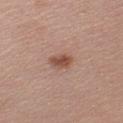Q: Was this lesion biopsied?
A: imaged on a skin check; not biopsied
Q: Patient demographics?
A: female, approximately 55 years of age
Q: Automated lesion metrics?
A: a lesion area of about 4.5 mm², an eccentricity of roughly 0.7, and a symmetry-axis asymmetry near 0.25; border irregularity of about 2.5 on a 0–10 scale and peripheral color asymmetry of about 1
Q: Lesion size?
A: ≈3 mm
Q: What is the anatomic site?
A: the left upper arm
Q: How was this image acquired?
A: ~15 mm crop, total-body skin-cancer survey